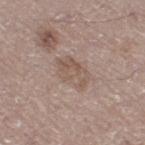biopsy_status: not biopsied; imaged during a skin examination